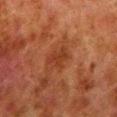The lesion was photographed on a routine skin check and not biopsied; there is no pathology result. The tile uses cross-polarized illumination. An algorithmic analysis of the crop reported a lesion area of about 5 mm², an outline eccentricity of about 0.65 (0 = round, 1 = elongated), and a symmetry-axis asymmetry near 0.25. It also reported a lesion color around L≈30 a*≈23 b*≈29 in CIELAB, about 6 CIELAB-L* units darker than the surrounding skin, and a lesion-to-skin contrast of about 6 (normalized; higher = more distinct). It also reported a border-irregularity index near 3/10 and internal color variation of about 1.5 on a 0–10 scale. The lesion's longest dimension is about 3 mm. A male subject, about 80 years old. Cropped from a total-body skin-imaging series; the visible field is about 15 mm. Located on the left lower leg.